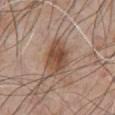Case summary:
• workup · no biopsy performed (imaged during a skin exam)
• patient · male, approximately 50 years of age
• lesion diameter · ≈4.5 mm
• anatomic site · the chest
• imaging modality · ~15 mm crop, total-body skin-cancer survey
• illumination · white-light